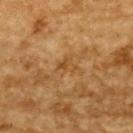Context: A 15 mm close-up extracted from a 3D total-body photography capture. The patient is a male about 85 years old or older. The lesion is on the back. Longest diameter approximately 2.5 mm. The total-body-photography lesion software estimated a color-variation rating of about 3/10 and a peripheral color-asymmetry measure near 1. The analysis additionally found a nevus-likeness score of about 0/100 and lesion-presence confidence of about 95/100. Captured under cross-polarized illumination.